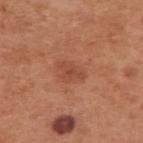Assessment:
No biopsy was performed on this lesion — it was imaged during a full skin examination and was not determined to be concerning.
Clinical summary:
About 3 mm across. The lesion-visualizer software estimated a lesion area of about 3 mm², an outline eccentricity of about 0.85 (0 = round, 1 = elongated), and two-axis asymmetry of about 0.5. The analysis additionally found an average lesion color of about L≈47 a*≈27 b*≈32 (CIELAB), a lesion–skin lightness drop of about 8, and a normalized border contrast of about 5.5. And it measured a nevus-likeness score of about 5/100. Cropped from a whole-body photographic skin survey; the tile spans about 15 mm. The patient is a male aged approximately 55. The tile uses white-light illumination. Located on the upper back.